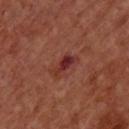No biopsy was performed on this lesion — it was imaged during a full skin examination and was not determined to be concerning.
A male subject in their mid-60s.
Captured under cross-polarized illumination.
The lesion is on the chest.
Approximately 3.5 mm at its widest.
A lesion tile, about 15 mm wide, cut from a 3D total-body photograph.